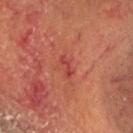Clinical impression: The lesion was tiled from a total-body skin photograph and was not biopsied. Acquisition and patient details: Captured under cross-polarized illumination. This image is a 15 mm lesion crop taken from a total-body photograph. On the head or neck. The subject is a male roughly 55 years of age.